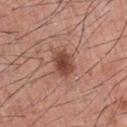The patient is a male aged 43–47.
Longest diameter approximately 3 mm.
Imaged with white-light lighting.
Located on the upper back.
Cropped from a whole-body photographic skin survey; the tile spans about 15 mm.